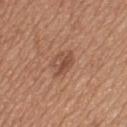follow-up: total-body-photography surveillance lesion; no biopsy | subject: male, approximately 65 years of age | lesion size: about 3.5 mm | lighting: white-light illumination | image source: 15 mm crop, total-body photography | site: the upper back.